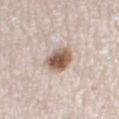| key | value |
|---|---|
| notes | no biopsy performed (imaged during a skin exam) |
| lesion diameter | ~3.5 mm (longest diameter) |
| subject | male, approximately 80 years of age |
| automated metrics | an area of roughly 9 mm² and a shape-asymmetry score of about 0.15 (0 = symmetric) |
| image | ~15 mm crop, total-body skin-cancer survey |
| site | the left lower leg |
| illumination | white-light illumination |